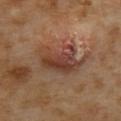The lesion was photographed on a routine skin check and not biopsied; there is no pathology result.
A roughly 15 mm field-of-view crop from a total-body skin photograph.
The lesion is on the mid back.
A female subject, aged around 55.